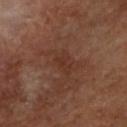The lesion was tiled from a total-body skin photograph and was not biopsied.